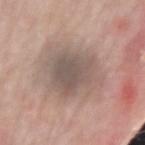notes: catalogued during a skin exam; not biopsied
imaging modality: ~15 mm tile from a whole-body skin photo
patient: male, aged 73–77
lighting: white-light illumination
body site: the right forearm
lesion size: ~7 mm (longest diameter)
TBP lesion metrics: a lesion area of about 24 mm², an outline eccentricity of about 0.75 (0 = round, 1 = elongated), and two-axis asymmetry of about 0.25; a mean CIELAB color near L≈54 a*≈13 b*≈21 and a lesion-to-skin contrast of about 7 (normalized; higher = more distinct); a border-irregularity rating of about 3/10, internal color variation of about 5 on a 0–10 scale, and a peripheral color-asymmetry measure near 1.5